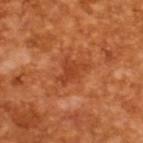Impression: The lesion was tiled from a total-body skin photograph and was not biopsied. Background: A 15 mm crop from a total-body photograph taken for skin-cancer surveillance. The total-body-photography lesion software estimated a footprint of about 6 mm², a shape eccentricity near 0.7, and a symmetry-axis asymmetry near 0.5. The software also gave a lesion–skin lightness drop of about 8. It also reported a color-variation rating of about 1.5/10 and a peripheral color-asymmetry measure near 0.5. And it measured a lesion-detection confidence of about 100/100. Measured at roughly 3.5 mm in maximum diameter. A male patient aged approximately 65.